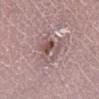Notes:
* notes: imaged on a skin check; not biopsied
* subject: male, in their 30s
* lighting: white-light
* site: the right lower leg
* diameter: ~4 mm (longest diameter)
* acquisition: ~15 mm tile from a whole-body skin photo